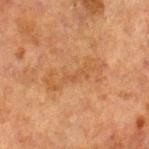Context:
The tile uses cross-polarized illumination. Measured at roughly 6.5 mm in maximum diameter. The subject is a male about 70 years old. A 15 mm crop from a total-body photograph taken for skin-cancer surveillance. The lesion is on the right lower leg.